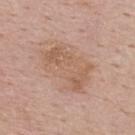Q: What is the anatomic site?
A: the upper back
Q: How was the tile lit?
A: white-light illumination
Q: Who is the patient?
A: male, about 55 years old
Q: What did automated image analysis measure?
A: a lesion area of about 21 mm², a shape eccentricity near 0.75, and two-axis asymmetry of about 0.25; a border-irregularity index near 3.5/10, a within-lesion color-variation index near 3.5/10, and a peripheral color-asymmetry measure near 1
Q: What is the imaging modality?
A: 15 mm crop, total-body photography